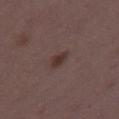Assessment: Imaged during a routine full-body skin examination; the lesion was not biopsied and no histopathology is available. Acquisition and patient details: A close-up tile cropped from a whole-body skin photograph, about 15 mm across. The subject is a female aged around 35. Captured under white-light illumination. From the right thigh.